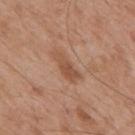- notes · imaged on a skin check; not biopsied
- location · the mid back
- subject · male, in their mid-50s
- image source · 15 mm crop, total-body photography
- tile lighting · white-light
- lesion size · ~4.5 mm (longest diameter)